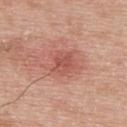biopsy_status: not biopsied; imaged during a skin examination
lesion_size:
  long_diameter_mm_approx: 2.5
site: upper back
lighting: white-light
patient:
  sex: male
  age_approx: 65
image:
  source: total-body photography crop
  field_of_view_mm: 15